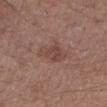Recorded during total-body skin imaging; not selected for excision or biopsy.
Longest diameter approximately 3.5 mm.
On the arm.
Captured under white-light illumination.
Automated tile analysis of the lesion measured an area of roughly 7.5 mm², an outline eccentricity of about 0.65 (0 = round, 1 = elongated), and a shape-asymmetry score of about 0.35 (0 = symmetric). The software also gave a within-lesion color-variation index near 2.5/10 and peripheral color asymmetry of about 1. The software also gave a classifier nevus-likeness of about 5/100 and a lesion-detection confidence of about 100/100.
A 15 mm close-up tile from a total-body photography series done for melanoma screening.
The subject is a female aged approximately 55.